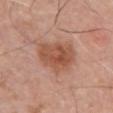Q: Was this lesion biopsied?
A: no biopsy performed (imaged during a skin exam)
Q: What are the patient's age and sex?
A: male, roughly 70 years of age
Q: What kind of image is this?
A: ~15 mm tile from a whole-body skin photo
Q: What is the lesion's diameter?
A: about 5.5 mm
Q: Where on the body is the lesion?
A: the chest
Q: What lighting was used for the tile?
A: white-light